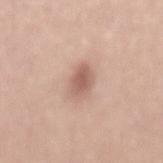Q: How was this image acquired?
A: 15 mm crop, total-body photography
Q: What are the patient's age and sex?
A: male, aged 48–52
Q: Lesion size?
A: about 3.5 mm
Q: What lighting was used for the tile?
A: white-light illumination
Q: What is the anatomic site?
A: the back